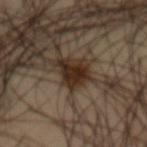Q: Was this lesion biopsied?
A: catalogued during a skin exam; not biopsied
Q: Patient demographics?
A: male, aged 38 to 42
Q: Lesion size?
A: ~4 mm (longest diameter)
Q: What lighting was used for the tile?
A: cross-polarized
Q: Where on the body is the lesion?
A: the front of the torso
Q: Automated lesion metrics?
A: a footprint of about 7.5 mm² and a shape-asymmetry score of about 0.35 (0 = symmetric); about 13 CIELAB-L* units darker than the surrounding skin and a normalized border contrast of about 13.5
Q: How was this image acquired?
A: ~15 mm crop, total-body skin-cancer survey